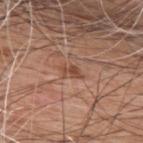notes = total-body-photography surveillance lesion; no biopsy.